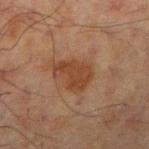This lesion was catalogued during total-body skin photography and was not selected for biopsy. The lesion is on the right upper arm. A male patient, about 70 years old. This image is a 15 mm lesion crop taken from a total-body photograph.